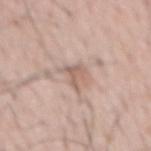| key | value |
|---|---|
| biopsy status | total-body-photography surveillance lesion; no biopsy |
| automated metrics | a lesion area of about 4 mm² and a shape-asymmetry score of about 0.45 (0 = symmetric) |
| subject | male, aged 63–67 |
| acquisition | ~15 mm tile from a whole-body skin photo |
| size | ~3 mm (longest diameter) |
| site | the mid back |